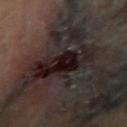Recorded during total-body skin imaging; not selected for excision or biopsy. The lesion's longest dimension is about 7.5 mm. The subject is a male roughly 65 years of age. Captured under cross-polarized illumination. On the right upper arm. A 15 mm crop from a total-body photograph taken for skin-cancer surveillance.